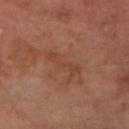| field | value |
|---|---|
| biopsy status | catalogued during a skin exam; not biopsied |
| size | ≈4.5 mm |
| lighting | cross-polarized illumination |
| image source | ~15 mm tile from a whole-body skin photo |
| location | the arm |
| patient | female, aged 63 to 67 |
| automated lesion analysis | a mean CIELAB color near L≈42 a*≈22 b*≈31, a lesion–skin lightness drop of about 6, and a normalized lesion–skin contrast near 5; a border-irregularity index near 8/10, a color-variation rating of about 0.5/10, and peripheral color asymmetry of about 0; an automated nevus-likeness rating near 0 out of 100 and a detector confidence of about 100 out of 100 that the crop contains a lesion |